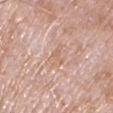Part of a total-body skin-imaging series; this lesion was reviewed on a skin check and was not flagged for biopsy. A male patient approximately 65 years of age. This is a white-light tile. From the chest. The recorded lesion diameter is about 3 mm. Cropped from a total-body skin-imaging series; the visible field is about 15 mm. An algorithmic analysis of the crop reported a mean CIELAB color near L≈64 a*≈19 b*≈30 and a normalized border contrast of about 5. And it measured a within-lesion color-variation index near 0.5/10 and a peripheral color-asymmetry measure near 0.